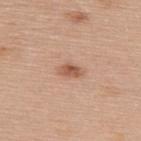Q: Is there a histopathology result?
A: catalogued during a skin exam; not biopsied
Q: What lighting was used for the tile?
A: white-light
Q: Automated lesion metrics?
A: roughly 11 lightness units darker than nearby skin and a normalized border contrast of about 7.5; a nevus-likeness score of about 85/100
Q: What is the lesion's diameter?
A: ≈3 mm
Q: What is the imaging modality?
A: 15 mm crop, total-body photography
Q: What are the patient's age and sex?
A: female, aged approximately 60
Q: Where on the body is the lesion?
A: the upper back